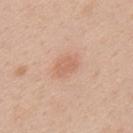No biopsy was performed on this lesion — it was imaged during a full skin examination and was not determined to be concerning. Automated image analysis of the tile measured a lesion area of about 4.5 mm², an outline eccentricity of about 0.75 (0 = round, 1 = elongated), and two-axis asymmetry of about 0.2. The software also gave an average lesion color of about L≈63 a*≈23 b*≈31 (CIELAB) and a lesion–skin lightness drop of about 7. From the upper back. A roughly 15 mm field-of-view crop from a total-body skin photograph. The recorded lesion diameter is about 3 mm. A male subject approximately 35 years of age.